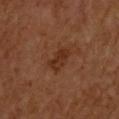* workup: catalogued during a skin exam; not biopsied
* diameter: ~3 mm (longest diameter)
* imaging modality: 15 mm crop, total-body photography
* patient: male, roughly 60 years of age
* anatomic site: the upper back
* lighting: cross-polarized illumination